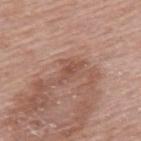Captured during whole-body skin photography for melanoma surveillance; the lesion was not biopsied. Imaged with white-light lighting. A female patient, aged around 60. The lesion is located on the upper back. The recorded lesion diameter is about 2.5 mm. A region of skin cropped from a whole-body photographic capture, roughly 15 mm wide.